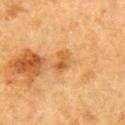Captured during whole-body skin photography for melanoma surveillance; the lesion was not biopsied.
About 3 mm across.
The lesion is on the right upper arm.
The subject is a male aged 83 or older.
Imaged with cross-polarized lighting.
A region of skin cropped from a whole-body photographic capture, roughly 15 mm wide.
The lesion-visualizer software estimated an area of roughly 4.5 mm², a shape eccentricity near 0.75, and a shape-asymmetry score of about 0.35 (0 = symmetric). The analysis additionally found a border-irregularity rating of about 3.5/10, a within-lesion color-variation index near 4.5/10, and radial color variation of about 1.5. And it measured a classifier nevus-likeness of about 0/100.